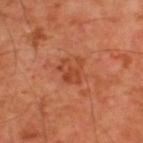Captured during whole-body skin photography for melanoma surveillance; the lesion was not biopsied.
The total-body-photography lesion software estimated a lesion area of about 6 mm². The analysis additionally found a border-irregularity rating of about 5/10, internal color variation of about 3 on a 0–10 scale, and a peripheral color-asymmetry measure near 1. The software also gave an automated nevus-likeness rating near 15 out of 100 and a lesion-detection confidence of about 100/100.
This image is a 15 mm lesion crop taken from a total-body photograph.
A male patient about 60 years old.
The lesion is on the upper back.
Measured at roughly 3 mm in maximum diameter.